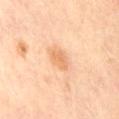The lesion was photographed on a routine skin check and not biopsied; there is no pathology result. The recorded lesion diameter is about 3 mm. The tile uses cross-polarized illumination. This image is a 15 mm lesion crop taken from a total-body photograph. A female subject, aged 48 to 52. The lesion-visualizer software estimated a lesion color around L≈70 a*≈22 b*≈39 in CIELAB and a normalized border contrast of about 6. It also reported a border-irregularity rating of about 1.5/10 and radial color variation of about 0.5. It also reported a classifier nevus-likeness of about 55/100 and a detector confidence of about 100 out of 100 that the crop contains a lesion. The lesion is on the chest.